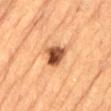Clinical summary: Cropped from a whole-body photographic skin survey; the tile spans about 15 mm. From the back. Measured at roughly 4.5 mm in maximum diameter. A male patient, in their mid-80s or older. The tile uses cross-polarized illumination.